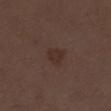Part of a total-body skin-imaging series; this lesion was reviewed on a skin check and was not flagged for biopsy. The lesion is located on the right lower leg. A female patient, aged 48 to 52. This is a white-light tile. Approximately 2.5 mm at its widest. A 15 mm crop from a total-body photograph taken for skin-cancer surveillance.